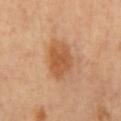site=the mid back; illumination=cross-polarized; image source=~15 mm tile from a whole-body skin photo; diameter=~4.5 mm (longest diameter); TBP lesion metrics=an automated nevus-likeness rating near 95 out of 100 and a lesion-detection confidence of about 100/100.